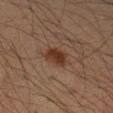workup: total-body-photography surveillance lesion; no biopsy | illumination: cross-polarized illumination | body site: the right forearm | size: ~3.5 mm (longest diameter) | patient: male, aged around 35 | image: ~15 mm crop, total-body skin-cancer survey.